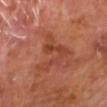{
  "biopsy_status": "not biopsied; imaged during a skin examination",
  "lighting": "cross-polarized",
  "image": {
    "source": "total-body photography crop",
    "field_of_view_mm": 15
  },
  "lesion_size": {
    "long_diameter_mm_approx": 5.5
  },
  "patient": {
    "sex": "male",
    "age_approx": 60
  },
  "site": "upper back"
}